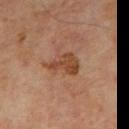Clinical impression: Captured during whole-body skin photography for melanoma surveillance; the lesion was not biopsied. Clinical summary: From the mid back. Captured under cross-polarized illumination. The lesion-visualizer software estimated a footprint of about 8.5 mm² and a shape eccentricity near 0.8. It also reported a lesion-to-skin contrast of about 7.5 (normalized; higher = more distinct). A 15 mm close-up extracted from a 3D total-body photography capture. A male subject, aged 68 to 72. About 4.5 mm across.